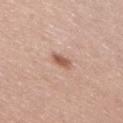notes = imaged on a skin check; not biopsied
acquisition = total-body-photography crop, ~15 mm field of view
image-analysis metrics = roughly 12 lightness units darker than nearby skin; a border-irregularity rating of about 3/10 and internal color variation of about 1.5 on a 0–10 scale; a classifier nevus-likeness of about 75/100 and a lesion-detection confidence of about 100/100
anatomic site = the leg
illumination = white-light
subject = female, aged around 40